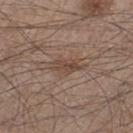An algorithmic analysis of the crop reported a lesion area of about 6 mm², an eccentricity of roughly 0.85, and a shape-asymmetry score of about 0.25 (0 = symmetric). And it measured a lesion color around L≈45 a*≈16 b*≈25 in CIELAB. And it measured a border-irregularity rating of about 3.5/10, a within-lesion color-variation index near 3/10, and radial color variation of about 1.
This image is a 15 mm lesion crop taken from a total-body photograph.
Imaged with white-light lighting.
The lesion is on the leg.
A male subject, aged 43 to 47.
Approximately 4 mm at its widest.